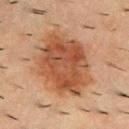  biopsy_status: not biopsied; imaged during a skin examination
  image:
    source: total-body photography crop
    field_of_view_mm: 15
  site: upper back
  lesion_size:
    long_diameter_mm_approx: 7.5
  lighting: cross-polarized
  patient:
    sex: male
    age_approx: 55